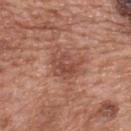* notes · total-body-photography surveillance lesion; no biopsy
* illumination · white-light
* imaging modality · 15 mm crop, total-body photography
* size · ≈4 mm
* TBP lesion metrics · a footprint of about 9.5 mm² and a shape-asymmetry score of about 0.25 (0 = symmetric); a lesion color around L≈49 a*≈24 b*≈28 in CIELAB, roughly 9 lightness units darker than nearby skin, and a normalized border contrast of about 6.5; a border-irregularity index near 3/10 and internal color variation of about 4.5 on a 0–10 scale
* patient · female, aged approximately 60
* body site · the upper back